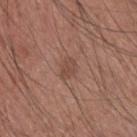| key | value |
|---|---|
| biopsy status | total-body-photography surveillance lesion; no biopsy |
| image source | ~15 mm tile from a whole-body skin photo |
| anatomic site | the upper back |
| tile lighting | white-light illumination |
| patient | male, aged approximately 35 |